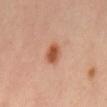biopsy status — imaged on a skin check; not biopsied | patient — male, aged 38 to 42 | image — ~15 mm crop, total-body skin-cancer survey | lighting — cross-polarized | site — the mid back.